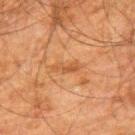No biopsy was performed on this lesion — it was imaged during a full skin examination and was not determined to be concerning.
On the upper back.
A lesion tile, about 15 mm wide, cut from a 3D total-body photograph.
The recorded lesion diameter is about 3 mm.
The subject is a male aged around 60.
An algorithmic analysis of the crop reported roughly 6 lightness units darker than nearby skin and a normalized border contrast of about 5.5. And it measured a detector confidence of about 100 out of 100 that the crop contains a lesion.